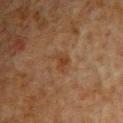follow-up: no biopsy performed (imaged during a skin exam)
subject: male, in their 50s
image source: ~15 mm crop, total-body skin-cancer survey
tile lighting: cross-polarized
body site: the upper back